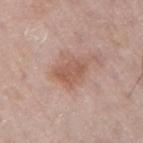On the arm. A lesion tile, about 15 mm wide, cut from a 3D total-body photograph. The patient is a male aged 63 to 67.